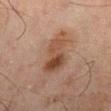The lesion was tiled from a total-body skin photograph and was not biopsied.
From the leg.
The tile uses cross-polarized illumination.
A close-up tile cropped from a whole-body skin photograph, about 15 mm across.
The patient is a male aged approximately 45.
Approximately 6 mm at its widest.